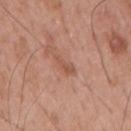{"biopsy_status": "not biopsied; imaged during a skin examination", "lighting": "white-light", "patient": {"sex": "male", "age_approx": 55}, "image": {"source": "total-body photography crop", "field_of_view_mm": 15}, "automated_metrics": {"area_mm2_approx": 3.0, "eccentricity": 0.9, "shape_asymmetry": 0.45, "vs_skin_darker_L": 8.0, "vs_skin_contrast_norm": 6.0, "nevus_likeness_0_100": 0, "lesion_detection_confidence_0_100": 100}, "lesion_size": {"long_diameter_mm_approx": 3.0}, "site": "mid back"}